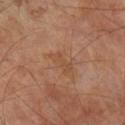Q: Is there a histopathology result?
A: catalogued during a skin exam; not biopsied
Q: Illumination type?
A: cross-polarized illumination
Q: What are the patient's age and sex?
A: male, approximately 70 years of age
Q: Where on the body is the lesion?
A: the right lower leg
Q: What is the lesion's diameter?
A: about 3.5 mm
Q: What kind of image is this?
A: ~15 mm tile from a whole-body skin photo